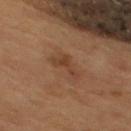The lesion was tiled from a total-body skin photograph and was not biopsied. The lesion is on the mid back. Approximately 4 mm at its widest. A region of skin cropped from a whole-body photographic capture, roughly 15 mm wide. A male patient, aged around 55. Captured under cross-polarized illumination. The total-body-photography lesion software estimated a footprint of about 5 mm² and two-axis asymmetry of about 0.6. It also reported an average lesion color of about L≈37 a*≈18 b*≈28 (CIELAB), about 7 CIELAB-L* units darker than the surrounding skin, and a normalized border contrast of about 6. The software also gave border irregularity of about 6.5 on a 0–10 scale and a within-lesion color-variation index near 1.5/10. And it measured an automated nevus-likeness rating near 0 out of 100 and a lesion-detection confidence of about 100/100.